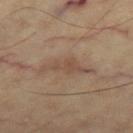No biopsy was performed on this lesion — it was imaged during a full skin examination and was not determined to be concerning. A male subject aged 63 to 67. The lesion is located on the right thigh. Cropped from a whole-body photographic skin survey; the tile spans about 15 mm.